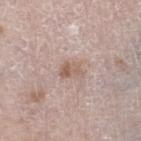Impression:
The lesion was photographed on a routine skin check and not biopsied; there is no pathology result.
Image and clinical context:
The patient is a male aged 78–82. This is a white-light tile. The total-body-photography lesion software estimated a lesion area of about 3 mm², an eccentricity of roughly 0.8, and two-axis asymmetry of about 0.35. The analysis additionally found border irregularity of about 3.5 on a 0–10 scale, a color-variation rating of about 3/10, and a peripheral color-asymmetry measure near 1. On the right lower leg. A 15 mm close-up extracted from a 3D total-body photography capture.